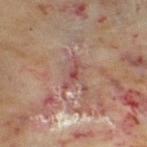{
  "biopsy_status": "not biopsied; imaged during a skin examination",
  "lesion_size": {
    "long_diameter_mm_approx": 3.5
  },
  "patient": {
    "sex": "female",
    "age_approx": 60
  },
  "image": {
    "source": "total-body photography crop",
    "field_of_view_mm": 15
  },
  "site": "left thigh"
}